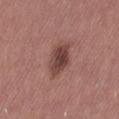biopsy status: total-body-photography surveillance lesion; no biopsy | automated lesion analysis: a shape-asymmetry score of about 0.25 (0 = symmetric); border irregularity of about 2.5 on a 0–10 scale, internal color variation of about 4 on a 0–10 scale, and radial color variation of about 1.5; a classifier nevus-likeness of about 55/100 and a lesion-detection confidence of about 100/100 | location: the lower back | patient: female, about 35 years old | illumination: white-light illumination | image source: ~15 mm tile from a whole-body skin photo | diameter: ~4 mm (longest diameter).